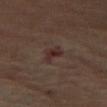biopsy status=imaged on a skin check; not biopsied | lesion diameter=about 3 mm | image=~15 mm crop, total-body skin-cancer survey | tile lighting=cross-polarized | subject=female, in their mid- to late 60s | TBP lesion metrics=a mean CIELAB color near L≈27 a*≈17 b*≈17, about 7 CIELAB-L* units darker than the surrounding skin, and a normalized lesion–skin contrast near 7.5 | location=the left leg.